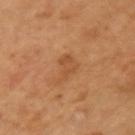{
  "biopsy_status": "not biopsied; imaged during a skin examination",
  "site": "left upper arm",
  "patient": {
    "sex": "female",
    "age_approx": 55
  },
  "image": {
    "source": "total-body photography crop",
    "field_of_view_mm": 15
  }
}